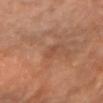Case summary:
– workup — total-body-photography surveillance lesion; no biopsy
– location — the left arm
– patient — female, in their mid- to late 60s
– acquisition — ~15 mm tile from a whole-body skin photo
– size — about 2.5 mm